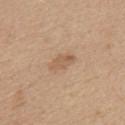biopsy_status: not biopsied; imaged during a skin examination
image:
  source: total-body photography crop
  field_of_view_mm: 15
lesion_size:
  long_diameter_mm_approx: 3.5
patient:
  sex: female
  age_approx: 45
site: front of the torso
automated_metrics:
  cielab_L: 58
  cielab_a: 18
  cielab_b: 32
  vs_skin_contrast_norm: 5.5
  color_variation_0_10: 2.5
  peripheral_color_asymmetry: 1.0